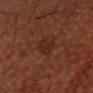Impression: The lesion was photographed on a routine skin check and not biopsied; there is no pathology result. Acquisition and patient details: Cropped from a whole-body photographic skin survey; the tile spans about 15 mm. The lesion-visualizer software estimated a lesion area of about 5.5 mm², an eccentricity of roughly 0.7, and a shape-asymmetry score of about 0.25 (0 = symmetric). And it measured lesion-presence confidence of about 100/100. Approximately 3.5 mm at its widest. The lesion is on the head or neck. A male subject roughly 65 years of age.